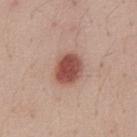Notes:
• follow-up · total-body-photography surveillance lesion; no biopsy
• image source · total-body-photography crop, ~15 mm field of view
• patient · male, about 35 years old
• site · the front of the torso